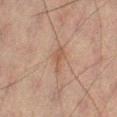Acquisition and patient details: The subject is a male aged approximately 60. Imaged with cross-polarized lighting. Automated image analysis of the tile measured border irregularity of about 4 on a 0–10 scale, a color-variation rating of about 1/10, and a peripheral color-asymmetry measure near 0.5. It also reported an automated nevus-likeness rating near 0 out of 100 and a detector confidence of about 100 out of 100 that the crop contains a lesion. The lesion's longest dimension is about 3.5 mm. Cropped from a total-body skin-imaging series; the visible field is about 15 mm. Located on the left leg.